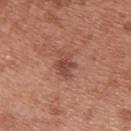Case summary:
– workup — catalogued during a skin exam; not biopsied
– anatomic site — the upper back
– subject — female, aged around 40
– imaging modality — total-body-photography crop, ~15 mm field of view